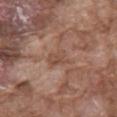The lesion was tiled from a total-body skin photograph and was not biopsied. Located on the back. A region of skin cropped from a whole-body photographic capture, roughly 15 mm wide. Imaged with white-light lighting. The patient is a male aged 73–77.